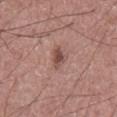Context: Automated tile analysis of the lesion measured a shape eccentricity near 0.7 and two-axis asymmetry of about 0.35. The analysis additionally found an average lesion color of about L≈49 a*≈21 b*≈23 (CIELAB). It also reported a border-irregularity rating of about 3/10, a within-lesion color-variation index near 2.5/10, and a peripheral color-asymmetry measure near 0.5. The subject is a male aged 58 to 62. About 2.5 mm across. Cropped from a whole-body photographic skin survey; the tile spans about 15 mm. Located on the abdomen.